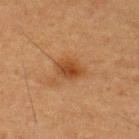biopsy status: no biopsy performed (imaged during a skin exam)
subject: male, in their mid- to late 30s
location: the upper back
imaging modality: 15 mm crop, total-body photography
size: about 3.5 mm
automated lesion analysis: a shape eccentricity near 0.75 and a symmetry-axis asymmetry near 0.25; a lesion color around L≈35 a*≈19 b*≈32 in CIELAB and about 8 CIELAB-L* units darker than the surrounding skin; a lesion-detection confidence of about 100/100